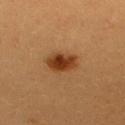The recorded lesion diameter is about 4 mm.
The lesion-visualizer software estimated a footprint of about 8.5 mm² and an outline eccentricity of about 0.7 (0 = round, 1 = elongated).
A roughly 15 mm field-of-view crop from a total-body skin photograph.
From the upper back.
The tile uses cross-polarized illumination.
A female patient roughly 25 years of age.